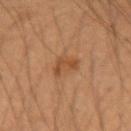{
  "biopsy_status": "not biopsied; imaged during a skin examination",
  "site": "right forearm",
  "patient": {
    "sex": "male",
    "age_approx": 35
  },
  "image": {
    "source": "total-body photography crop",
    "field_of_view_mm": 15
  }
}